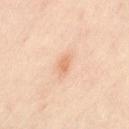Case summary:
• workup · imaged on a skin check; not biopsied
• tile lighting · cross-polarized illumination
• patient · female, roughly 40 years of age
• site · the right leg
• acquisition · ~15 mm crop, total-body skin-cancer survey
• lesion size · ~2.5 mm (longest diameter)